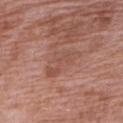follow-up: total-body-photography surveillance lesion; no biopsy
location: the right upper arm
image: total-body-photography crop, ~15 mm field of view
subject: female, in their 70s